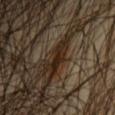biopsy status = catalogued during a skin exam; not biopsied | image source = 15 mm crop, total-body photography | patient = male, aged 43 to 47 | site = the right upper arm.